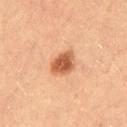Part of a total-body skin-imaging series; this lesion was reviewed on a skin check and was not flagged for biopsy.
Cropped from a whole-body photographic skin survey; the tile spans about 15 mm.
About 3.5 mm across.
The lesion-visualizer software estimated a classifier nevus-likeness of about 100/100.
A female subject roughly 20 years of age.
The tile uses cross-polarized illumination.
Located on the left thigh.